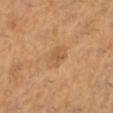biopsy status = imaged on a skin check; not biopsied
acquisition = ~15 mm crop, total-body skin-cancer survey
subject = female, roughly 55 years of age
location = the left lower leg
automated lesion analysis = a border-irregularity rating of about 2/10 and a within-lesion color-variation index near 2.5/10; a classifier nevus-likeness of about 0/100 and lesion-presence confidence of about 100/100
lesion diameter = ~3.5 mm (longest diameter)
tile lighting = cross-polarized